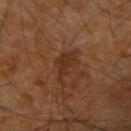Part of a total-body skin-imaging series; this lesion was reviewed on a skin check and was not flagged for biopsy. On the arm. This image is a 15 mm lesion crop taken from a total-body photograph. A male subject, in their 60s.